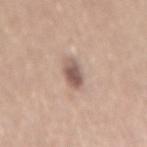Q: Was this lesion biopsied?
A: total-body-photography surveillance lesion; no biopsy
Q: How was the tile lit?
A: white-light illumination
Q: What is the imaging modality?
A: ~15 mm tile from a whole-body skin photo
Q: What is the lesion's diameter?
A: ~3.5 mm (longest diameter)
Q: What is the anatomic site?
A: the mid back
Q: Patient demographics?
A: female, aged 63 to 67
Q: Automated lesion metrics?
A: a mean CIELAB color near L≈56 a*≈17 b*≈23 and a lesion-to-skin contrast of about 9 (normalized; higher = more distinct); a border-irregularity index near 2/10, a within-lesion color-variation index near 4.5/10, and a peripheral color-asymmetry measure near 1.5